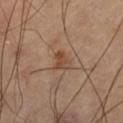{"image": {"source": "total-body photography crop", "field_of_view_mm": 15}, "lighting": "cross-polarized", "patient": {"sex": "male", "age_approx": 60}, "site": "left thigh"}